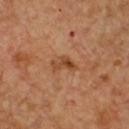Context: The total-body-photography lesion software estimated a footprint of about 3.5 mm² and an outline eccentricity of about 0.85 (0 = round, 1 = elongated). The software also gave a mean CIELAB color near L≈45 a*≈24 b*≈36 and roughly 9 lightness units darker than nearby skin. The analysis additionally found internal color variation of about 1.5 on a 0–10 scale and a peripheral color-asymmetry measure near 0.5. The software also gave a nevus-likeness score of about 0/100. The tile uses cross-polarized illumination. Measured at roughly 3 mm in maximum diameter. A region of skin cropped from a whole-body photographic capture, roughly 15 mm wide. A male subject about 50 years old. The lesion is on the chest.